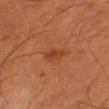Q: Is there a histopathology result?
A: no biopsy performed (imaged during a skin exam)
Q: Who is the patient?
A: male, approximately 50 years of age
Q: How was the tile lit?
A: cross-polarized illumination
Q: What did automated image analysis measure?
A: an area of roughly 3 mm², a shape eccentricity near 0.85, and a shape-asymmetry score of about 0.25 (0 = symmetric)
Q: What kind of image is this?
A: ~15 mm crop, total-body skin-cancer survey
Q: What is the anatomic site?
A: the leg